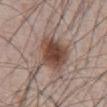Impression:
Recorded during total-body skin imaging; not selected for excision or biopsy.
Context:
A 15 mm close-up extracted from a 3D total-body photography capture. Located on the abdomen. The patient is a male aged around 65.